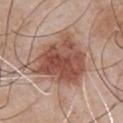This lesion was catalogued during total-body skin photography and was not selected for biopsy. This is a white-light tile. The lesion is on the chest. Longest diameter approximately 7 mm. A male patient, roughly 50 years of age. A roughly 15 mm field-of-view crop from a total-body skin photograph. Automated tile analysis of the lesion measured a normalized border contrast of about 9.5.